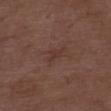The lesion was tiled from a total-body skin photograph and was not biopsied.
Cropped from a total-body skin-imaging series; the visible field is about 15 mm.
A male patient roughly 50 years of age.
This is a white-light tile.